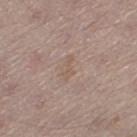Assessment: Captured during whole-body skin photography for melanoma surveillance; the lesion was not biopsied. Image and clinical context: On the left thigh. A female patient aged 48 to 52. Measured at roughly 3 mm in maximum diameter. The tile uses white-light illumination. A close-up tile cropped from a whole-body skin photograph, about 15 mm across.